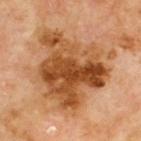No biopsy was performed on this lesion — it was imaged during a full skin examination and was not determined to be concerning. The lesion is located on the back. Captured under cross-polarized illumination. Measured at roughly 8.5 mm in maximum diameter. The subject is a male approximately 70 years of age. Automated image analysis of the tile measured a border-irregularity index near 4.5/10. A region of skin cropped from a whole-body photographic capture, roughly 15 mm wide.